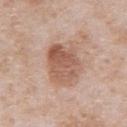Impression:
Recorded during total-body skin imaging; not selected for excision or biopsy.
Acquisition and patient details:
A close-up tile cropped from a whole-body skin photograph, about 15 mm across. The lesion is located on the abdomen. The patient is a male aged around 65.